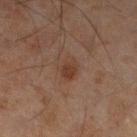The lesion was photographed on a routine skin check and not biopsied; there is no pathology result. The total-body-photography lesion software estimated an area of roughly 5 mm², an outline eccentricity of about 0.65 (0 = round, 1 = elongated), and a symmetry-axis asymmetry near 0.25. The software also gave an average lesion color of about L≈30 a*≈16 b*≈23 (CIELAB), about 6 CIELAB-L* units darker than the surrounding skin, and a normalized lesion–skin contrast near 6.5. It also reported a color-variation rating of about 2.5/10 and radial color variation of about 1. The software also gave an automated nevus-likeness rating near 55 out of 100 and a lesion-detection confidence of about 100/100. Imaged with cross-polarized lighting. A male patient, roughly 45 years of age. About 2.5 mm across. A lesion tile, about 15 mm wide, cut from a 3D total-body photograph. The lesion is located on the left lower leg.